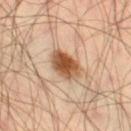Q: Was a biopsy performed?
A: no biopsy performed (imaged during a skin exam)
Q: What is the anatomic site?
A: the right thigh
Q: What is the lesion's diameter?
A: about 4 mm
Q: Patient demographics?
A: male, in their mid-50s
Q: What lighting was used for the tile?
A: cross-polarized
Q: What is the imaging modality?
A: 15 mm crop, total-body photography